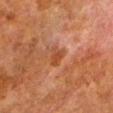Clinical summary: This is a cross-polarized tile. A 15 mm crop from a total-body photograph taken for skin-cancer surveillance. From the left lower leg. A male subject aged around 80.